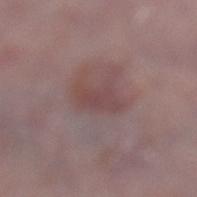The lesion is located on the left lower leg. A 15 mm close-up tile from a total-body photography series done for melanoma screening. A male subject, in their mid- to late 70s. The tile uses white-light illumination.